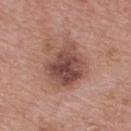follow-up: no biopsy performed (imaged during a skin exam) | location: the upper back | imaging modality: ~15 mm tile from a whole-body skin photo | patient: male, in their mid-60s | TBP lesion metrics: a lesion area of about 22 mm², an eccentricity of roughly 0.45, and two-axis asymmetry of about 0.25; roughly 12 lightness units darker than nearby skin and a lesion-to-skin contrast of about 8.5 (normalized; higher = more distinct).